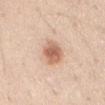The lesion was photographed on a routine skin check and not biopsied; there is no pathology result.
This is a white-light tile.
The patient is a male roughly 35 years of age.
From the abdomen.
The recorded lesion diameter is about 3.5 mm.
This image is a 15 mm lesion crop taken from a total-body photograph.